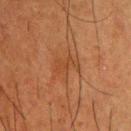Q: Is there a histopathology result?
A: no biopsy performed (imaged during a skin exam)
Q: Who is the patient?
A: male, about 35 years old
Q: What is the imaging modality?
A: ~15 mm crop, total-body skin-cancer survey
Q: What is the anatomic site?
A: the back